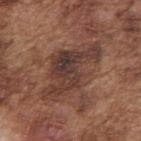Part of a total-body skin-imaging series; this lesion was reviewed on a skin check and was not flagged for biopsy. This image is a 15 mm lesion crop taken from a total-body photograph. A male patient, roughly 75 years of age. This is a white-light tile. The lesion is located on the right upper arm. Approximately 8.5 mm at its widest.